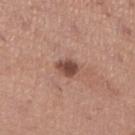Assessment:
Part of a total-body skin-imaging series; this lesion was reviewed on a skin check and was not flagged for biopsy.
Clinical summary:
This is a white-light tile. A female patient, in their mid- to late 60s. On the right lower leg. Automated image analysis of the tile measured a mean CIELAB color near L≈47 a*≈22 b*≈25 and about 13 CIELAB-L* units darker than the surrounding skin. And it measured internal color variation of about 2.5 on a 0–10 scale and radial color variation of about 1. The software also gave an automated nevus-likeness rating near 65 out of 100. A 15 mm crop from a total-body photograph taken for skin-cancer surveillance.